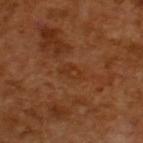patient: male, aged 63 to 67 | diameter: ≈3 mm | tile lighting: cross-polarized | acquisition: ~15 mm tile from a whole-body skin photo.